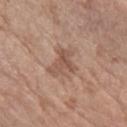The lesion was photographed on a routine skin check and not biopsied; there is no pathology result. A region of skin cropped from a whole-body photographic capture, roughly 15 mm wide. From the left forearm. A female subject about 75 years old. Captured under white-light illumination.